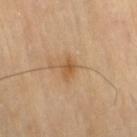Q: Was a biopsy performed?
A: catalogued during a skin exam; not biopsied
Q: Patient demographics?
A: female, aged around 70
Q: What kind of image is this?
A: ~15 mm crop, total-body skin-cancer survey
Q: What is the anatomic site?
A: the right thigh